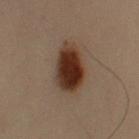| feature | finding |
|---|---|
| anatomic site | the left upper arm |
| subject | male, aged around 55 |
| image | ~15 mm tile from a whole-body skin photo |
| automated lesion analysis | an area of roughly 14 mm² and a symmetry-axis asymmetry near 0.15; a border-irregularity rating of about 1.5/10, a within-lesion color-variation index near 5/10, and a peripheral color-asymmetry measure near 1.5; an automated nevus-likeness rating near 100 out of 100 |